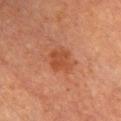  automated_metrics:
    area_mm2_approx: 7.0
    eccentricity: 0.5
  patient:
    sex: female
    age_approx: 60
  site: front of the torso
  image:
    source: total-body photography crop
    field_of_view_mm: 15